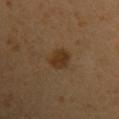Part of a total-body skin-imaging series; this lesion was reviewed on a skin check and was not flagged for biopsy. A female subject, approximately 35 years of age. On the left upper arm. Longest diameter approximately 3 mm. A 15 mm close-up extracted from a 3D total-body photography capture. Imaged with cross-polarized lighting.